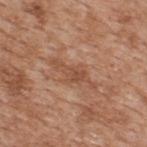Automated image analysis of the tile measured a footprint of about 7.5 mm², an eccentricity of roughly 0.95, and a shape-asymmetry score of about 0.35 (0 = symmetric). And it measured about 8 CIELAB-L* units darker than the surrounding skin and a normalized border contrast of about 5.5. And it measured a border-irregularity index near 6/10 and a color-variation rating of about 2.5/10. The analysis additionally found an automated nevus-likeness rating near 0 out of 100. A male patient, aged approximately 65. Longest diameter approximately 5.5 mm. From the back. A 15 mm close-up tile from a total-body photography series done for melanoma screening. Captured under white-light illumination.